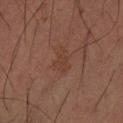  biopsy_status: not biopsied; imaged during a skin examination
  lighting: cross-polarized
  lesion_size:
    long_diameter_mm_approx: 3.0
  patient:
    sex: male
    age_approx: 50
  site: left forearm
  automated_metrics:
    cielab_L: 34
    cielab_a: 19
    cielab_b: 24
    vs_skin_darker_L: 4.0
    border_irregularity_0_10: 4.5
    color_variation_0_10: 0.5
    peripheral_color_asymmetry: 0.0
    nevus_likeness_0_100: 0
  image:
    source: total-body photography crop
    field_of_view_mm: 15